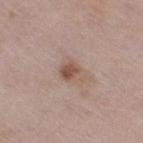Findings:
- biopsy status — no biopsy performed (imaged during a skin exam)
- body site — the right thigh
- imaging modality — 15 mm crop, total-body photography
- subject — female, aged 38 to 42
- size — about 3 mm